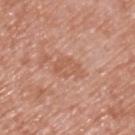notes = no biopsy performed (imaged during a skin exam)
subject = male, aged approximately 50
imaging modality = ~15 mm crop, total-body skin-cancer survey
location = the upper back
diameter = ~3.5 mm (longest diameter)
automated lesion analysis = a lesion color around L≈57 a*≈24 b*≈32 in CIELAB, roughly 7 lightness units darker than nearby skin, and a normalized border contrast of about 5
lighting = white-light illumination